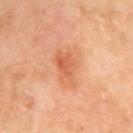biopsy status: total-body-photography surveillance lesion; no biopsy | subject: female, aged around 55 | lighting: cross-polarized illumination | automated metrics: an area of roughly 9 mm² and an outline eccentricity of about 0.8 (0 = round, 1 = elongated); a mean CIELAB color near L≈64 a*≈29 b*≈41, roughly 9 lightness units darker than nearby skin, and a normalized lesion–skin contrast near 6; border irregularity of about 3.5 on a 0–10 scale, a color-variation rating of about 5/10, and a peripheral color-asymmetry measure near 2 | lesion size: about 4.5 mm | image: total-body-photography crop, ~15 mm field of view | site: the arm.